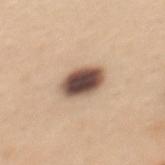Image and clinical context: On the mid back. A 15 mm close-up extracted from a 3D total-body photography capture. Longest diameter approximately 4 mm. A female subject, about 30 years old.